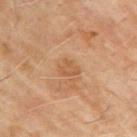automated metrics — a border-irregularity index near 3/10, a within-lesion color-variation index near 3/10, and peripheral color asymmetry of about 1.5; an automated nevus-likeness rating near 0 out of 100 and a detector confidence of about 100 out of 100 that the crop contains a lesion
location — the arm
patient — male, aged approximately 65
image source — ~15 mm crop, total-body skin-cancer survey
diameter — ≈2.5 mm
lighting — cross-polarized